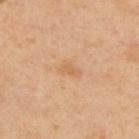Impression:
The lesion was photographed on a routine skin check and not biopsied; there is no pathology result.